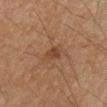Impression:
Captured during whole-body skin photography for melanoma surveillance; the lesion was not biopsied.
Image and clinical context:
A 15 mm close-up tile from a total-body photography series done for melanoma screening. The patient is a male aged approximately 55. From the left upper arm.